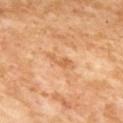The lesion was tiled from a total-body skin photograph and was not biopsied.
The subject is a female roughly 60 years of age.
Imaged with cross-polarized lighting.
This image is a 15 mm lesion crop taken from a total-body photograph.
About 3.5 mm across.
The lesion is located on the upper back.
The total-body-photography lesion software estimated a shape eccentricity near 0.95. The analysis additionally found a classifier nevus-likeness of about 0/100 and a lesion-detection confidence of about 100/100.